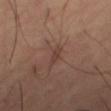notes = imaged on a skin check; not biopsied
patient = male, about 65 years old
diameter = about 3 mm
illumination = cross-polarized
acquisition = ~15 mm tile from a whole-body skin photo
automated metrics = an area of roughly 2.5 mm² and an eccentricity of roughly 0.95
body site = the leg